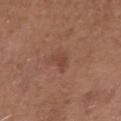size = ≈2.5 mm | illumination = white-light | body site = the head or neck | subject = male, approximately 70 years of age | acquisition = ~15 mm tile from a whole-body skin photo | automated metrics = a lesion area of about 3 mm², a shape eccentricity near 0.8, and two-axis asymmetry of about 0.4; about 7 CIELAB-L* units darker than the surrounding skin and a normalized border contrast of about 5.5; a nevus-likeness score of about 5/100 and a lesion-detection confidence of about 100/100.